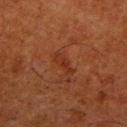Assessment:
This lesion was catalogued during total-body skin photography and was not selected for biopsy.
Background:
Cropped from a whole-body photographic skin survey; the tile spans about 15 mm. Imaged with cross-polarized lighting. From the right lower leg. Longest diameter approximately 3 mm. The patient is a male about 80 years old.